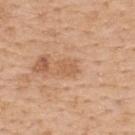{
  "biopsy_status": "not biopsied; imaged during a skin examination",
  "site": "upper back",
  "lesion_size": {
    "long_diameter_mm_approx": 2.5
  },
  "automated_metrics": {
    "cielab_L": 60,
    "cielab_a": 22,
    "cielab_b": 36,
    "vs_skin_darker_L": 7.0,
    "vs_skin_contrast_norm": 5.0,
    "nevus_likeness_0_100": 0,
    "lesion_detection_confidence_0_100": 100
  },
  "image": {
    "source": "total-body photography crop",
    "field_of_view_mm": 15
  },
  "patient": {
    "sex": "female",
    "age_approx": 45
  },
  "lighting": "white-light"
}